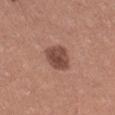No biopsy was performed on this lesion — it was imaged during a full skin examination and was not determined to be concerning. Approximately 3 mm at its widest. The subject is a female aged approximately 40. The tile uses white-light illumination. On the right thigh. A 15 mm crop from a total-body photograph taken for skin-cancer surveillance.